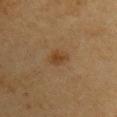Background: The patient is a female aged 53 to 57. Cropped from a total-body skin-imaging series; the visible field is about 15 mm. The lesion is on the right upper arm.